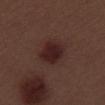This lesion was catalogued during total-body skin photography and was not selected for biopsy. A male subject aged 68 to 72. Located on the leg. Cropped from a total-body skin-imaging series; the visible field is about 15 mm.